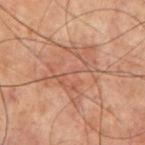Recorded during total-body skin imaging; not selected for excision or biopsy.